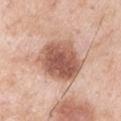workup — no biopsy performed (imaged during a skin exam) | anatomic site — the arm | size — about 5.5 mm | lighting — white-light illumination | patient — male, aged 53 to 57 | imaging modality — total-body-photography crop, ~15 mm field of view.